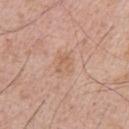The lesion was photographed on a routine skin check and not biopsied; there is no pathology result. Approximately 3 mm at its widest. A roughly 15 mm field-of-view crop from a total-body skin photograph. Automated image analysis of the tile measured a footprint of about 6 mm², an eccentricity of roughly 0.55, and two-axis asymmetry of about 0.25. It also reported border irregularity of about 2.5 on a 0–10 scale and a within-lesion color-variation index near 3.5/10. A male subject in their mid-60s. The lesion is located on the arm. This is a white-light tile.